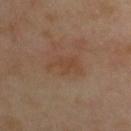Part of a total-body skin-imaging series; this lesion was reviewed on a skin check and was not flagged for biopsy.
This image is a 15 mm lesion crop taken from a total-body photograph.
A female patient about 40 years old.
An algorithmic analysis of the crop reported a lesion area of about 8 mm² and an outline eccentricity of about 0.85 (0 = round, 1 = elongated). And it measured a lesion color around L≈45 a*≈18 b*≈30 in CIELAB and a normalized border contrast of about 5.5.
From the upper back.